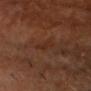– biopsy status: no biopsy performed (imaged during a skin exam)